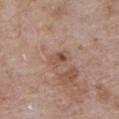Recorded during total-body skin imaging; not selected for excision or biopsy.
A roughly 15 mm field-of-view crop from a total-body skin photograph.
The lesion is on the chest.
The subject is a male aged 78–82.